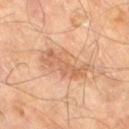• workup · no biopsy performed (imaged during a skin exam)
• lighting · cross-polarized
• imaging modality · ~15 mm crop, total-body skin-cancer survey
• image-analysis metrics · a classifier nevus-likeness of about 10/100 and a lesion-detection confidence of about 100/100
• subject · male, aged around 70
• site · the right thigh